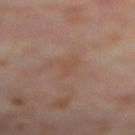Case summary:
* biopsy status · no biopsy performed (imaged during a skin exam)
* imaging modality · ~15 mm tile from a whole-body skin photo
* site · the right thigh
* TBP lesion metrics · an automated nevus-likeness rating near 0 out of 100 and a lesion-detection confidence of about 100/100
* subject · female, aged 53 to 57
* lesion diameter · ≈3 mm
* tile lighting · cross-polarized